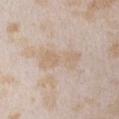Clinical impression: Part of a total-body skin-imaging series; this lesion was reviewed on a skin check and was not flagged for biopsy. Image and clinical context: Located on the front of the torso. A 15 mm close-up extracted from a 3D total-body photography capture. A female patient aged approximately 25.